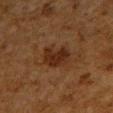Notes:
• biopsy status · catalogued during a skin exam; not biopsied
• lesion size · about 4 mm
• illumination · cross-polarized
• anatomic site · the right upper arm
• image source · ~15 mm crop, total-body skin-cancer survey
• patient · male, roughly 60 years of age
• TBP lesion metrics · a footprint of about 8.5 mm², an outline eccentricity of about 0.75 (0 = round, 1 = elongated), and two-axis asymmetry of about 0.3